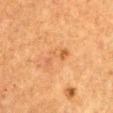Assessment:
Part of a total-body skin-imaging series; this lesion was reviewed on a skin check and was not flagged for biopsy.